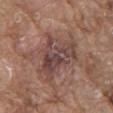• notes · imaged on a skin check; not biopsied
• tile lighting · white-light
• anatomic site · the back
• automated lesion analysis · a border-irregularity rating of about 5.5/10, internal color variation of about 6.5 on a 0–10 scale, and a peripheral color-asymmetry measure near 2
• lesion size · about 6.5 mm
• subject · male, aged around 80
• image · total-body-photography crop, ~15 mm field of view